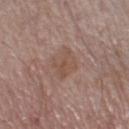patient=male, approximately 60 years of age
site=the left lower leg
imaging modality=~15 mm tile from a whole-body skin photo